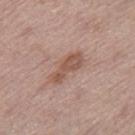Captured during whole-body skin photography for melanoma surveillance; the lesion was not biopsied. The tile uses white-light illumination. A female subject aged around 65. From the right thigh. A close-up tile cropped from a whole-body skin photograph, about 15 mm across. An algorithmic analysis of the crop reported a mean CIELAB color near L≈53 a*≈20 b*≈26 and roughly 9 lightness units darker than nearby skin. It also reported internal color variation of about 2.5 on a 0–10 scale and radial color variation of about 1. It also reported a classifier nevus-likeness of about 0/100 and lesion-presence confidence of about 100/100.